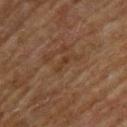follow-up = imaged on a skin check; not biopsied
lighting = cross-polarized illumination
lesion size = about 2.5 mm
imaging modality = ~15 mm tile from a whole-body skin photo
site = the upper back
patient = male, aged around 65